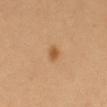Q: Was a biopsy performed?
A: catalogued during a skin exam; not biopsied
Q: How was this image acquired?
A: ~15 mm tile from a whole-body skin photo
Q: Where on the body is the lesion?
A: the mid back
Q: What did automated image analysis measure?
A: a lesion color around L≈56 a*≈22 b*≈40 in CIELAB, about 10 CIELAB-L* units darker than the surrounding skin, and a normalized border contrast of about 7.5; a nevus-likeness score of about 95/100 and a lesion-detection confidence of about 100/100
Q: How large is the lesion?
A: ~2 mm (longest diameter)
Q: Who is the patient?
A: female, aged approximately 55
Q: Illumination type?
A: cross-polarized illumination